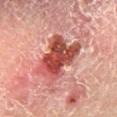Notes:
• notes · total-body-photography surveillance lesion; no biopsy
• patient · male, about 55 years old
• image-analysis metrics · an area of roughly 19 mm², an outline eccentricity of about 0.65 (0 = round, 1 = elongated), and a shape-asymmetry score of about 0.3 (0 = symmetric); border irregularity of about 4 on a 0–10 scale, a within-lesion color-variation index near 7.5/10, and peripheral color asymmetry of about 2.5
• acquisition · ~15 mm tile from a whole-body skin photo
• body site · the right lower leg
• diameter · about 5.5 mm